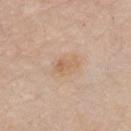Findings:
* follow-up — total-body-photography surveillance lesion; no biopsy
* imaging modality — 15 mm crop, total-body photography
* illumination — white-light illumination
* location — the chest
* lesion size — about 3 mm
* patient — male, in their mid-60s
* automated lesion analysis — a footprint of about 5 mm², an eccentricity of roughly 0.8, and a symmetry-axis asymmetry near 0.35; a border-irregularity rating of about 4/10, a color-variation rating of about 2.5/10, and radial color variation of about 0.5; a classifier nevus-likeness of about 0/100 and lesion-presence confidence of about 100/100